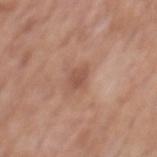anatomic site: the mid back
patient: male, about 70 years old
image-analysis metrics: a mean CIELAB color near L≈52 a*≈22 b*≈29, a lesion–skin lightness drop of about 9, and a normalized border contrast of about 6; border irregularity of about 2.5 on a 0–10 scale, internal color variation of about 2 on a 0–10 scale, and radial color variation of about 0.5; a classifier nevus-likeness of about 0/100 and lesion-presence confidence of about 100/100
imaging modality: 15 mm crop, total-body photography